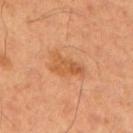Assessment: Part of a total-body skin-imaging series; this lesion was reviewed on a skin check and was not flagged for biopsy. Context: A male patient approximately 55 years of age. The tile uses cross-polarized illumination. About 4 mm across. From the arm. Automated image analysis of the tile measured a border-irregularity index near 4/10, a within-lesion color-variation index near 3/10, and peripheral color asymmetry of about 1. And it measured an automated nevus-likeness rating near 5 out of 100 and a detector confidence of about 100 out of 100 that the crop contains a lesion. A lesion tile, about 15 mm wide, cut from a 3D total-body photograph.